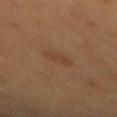* biopsy status — imaged on a skin check; not biopsied
* subject — male, aged around 50
* automated metrics — lesion-presence confidence of about 100/100
* illumination — cross-polarized illumination
* size — about 3.5 mm
* image — ~15 mm crop, total-body skin-cancer survey
* site — the mid back